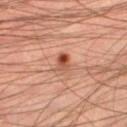<lesion>
<biopsy_status>not biopsied; imaged during a skin examination</biopsy_status>
<lesion_size>
  <long_diameter_mm_approx>2.5</long_diameter_mm_approx>
</lesion_size>
<image>
  <source>total-body photography crop</source>
  <field_of_view_mm>15</field_of_view_mm>
</image>
<site>left thigh</site>
<lighting>cross-polarized</lighting>
<patient>
  <sex>male</sex>
  <age_approx>45</age_approx>
</patient>
<automated_metrics>
  <area_mm2_approx>3.5</area_mm2_approx>
  <eccentricity>0.8</eccentricity>
  <shape_asymmetry>0.2</shape_asymmetry>
  <vs_skin_darker_L>12.0</vs_skin_darker_L>
  <vs_skin_contrast_norm>9.0</vs_skin_contrast_norm>
</automated_metrics>
</lesion>